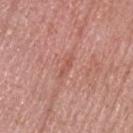biopsy_status: not biopsied; imaged during a skin examination
site: head or neck
lighting: white-light
patient:
  sex: male
  age_approx: 50
lesion_size:
  long_diameter_mm_approx: 2.5
image:
  source: total-body photography crop
  field_of_view_mm: 15